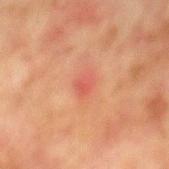<lesion>
<lighting>cross-polarized</lighting>
<image>
  <source>total-body photography crop</source>
  <field_of_view_mm>15</field_of_view_mm>
</image>
<site>mid back</site>
<patient>
  <sex>male</sex>
  <age_approx>75</age_approx>
</patient>
<automated_metrics>
  <area_mm2_approx>4.0</area_mm2_approx>
  <eccentricity>0.65</eccentricity>
  <shape_asymmetry>0.2</shape_asymmetry>
</automated_metrics>
<lesion_size>
  <long_diameter_mm_approx>2.5</long_diameter_mm_approx>
</lesion_size>
</lesion>